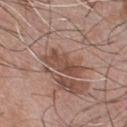Part of a total-body skin-imaging series; this lesion was reviewed on a skin check and was not flagged for biopsy.
Captured under white-light illumination.
Approximately 5.5 mm at its widest.
On the chest.
Automated tile analysis of the lesion measured a nevus-likeness score of about 15/100 and a detector confidence of about 95 out of 100 that the crop contains a lesion.
The subject is a male approximately 45 years of age.
A 15 mm crop from a total-body photograph taken for skin-cancer surveillance.